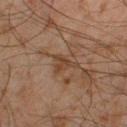Clinical impression: Imaged during a routine full-body skin examination; the lesion was not biopsied and no histopathology is available. Image and clinical context: This is a cross-polarized tile. The lesion is on the right lower leg. A male patient, in their mid- to late 40s. A 15 mm close-up tile from a total-body photography series done for melanoma screening. The total-body-photography lesion software estimated an area of roughly 4 mm² and an outline eccentricity of about 0.65 (0 = round, 1 = elongated). The analysis additionally found a mean CIELAB color near L≈33 a*≈15 b*≈25, about 6 CIELAB-L* units darker than the surrounding skin, and a normalized lesion–skin contrast near 6. And it measured a border-irregularity index near 6.5/10, internal color variation of about 1.5 on a 0–10 scale, and radial color variation of about 0.5.